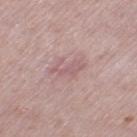Captured during whole-body skin photography for melanoma surveillance; the lesion was not biopsied.
A female patient, aged 38–42.
The lesion-visualizer software estimated an average lesion color of about L≈58 a*≈21 b*≈18 (CIELAB) and a lesion-to-skin contrast of about 5 (normalized; higher = more distinct). It also reported a border-irregularity rating of about 8/10 and a peripheral color-asymmetry measure near 0.
Located on the left thigh.
The tile uses white-light illumination.
A close-up tile cropped from a whole-body skin photograph, about 15 mm across.
About 3.5 mm across.